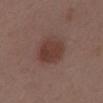Clinical impression:
No biopsy was performed on this lesion — it was imaged during a full skin examination and was not determined to be concerning.
Background:
The lesion's longest dimension is about 3.5 mm. A male patient, in their 50s. Captured under white-light illumination. Automated image analysis of the tile measured a lesion area of about 11 mm², a shape eccentricity near 0.35, and a symmetry-axis asymmetry near 0.1. And it measured a mean CIELAB color near L≈38 a*≈20 b*≈23, roughly 8 lightness units darker than nearby skin, and a normalized lesion–skin contrast near 8. The software also gave border irregularity of about 1 on a 0–10 scale and a within-lesion color-variation index near 2.5/10. It also reported an automated nevus-likeness rating near 80 out of 100. A roughly 15 mm field-of-view crop from a total-body skin photograph. From the back.